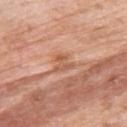Findings:
• patient: female, aged 73 to 77
• anatomic site: the upper back
• image source: 15 mm crop, total-body photography
• illumination: white-light